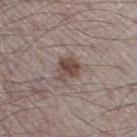| field | value |
|---|---|
| notes | catalogued during a skin exam; not biopsied |
| subject | male, aged around 50 |
| image source | ~15 mm tile from a whole-body skin photo |
| tile lighting | white-light |
| site | the right thigh |